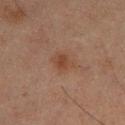Recorded during total-body skin imaging; not selected for excision or biopsy.
The lesion is located on the left lower leg.
The subject is a male aged 63–67.
A 15 mm crop from a total-body photograph taken for skin-cancer surveillance.